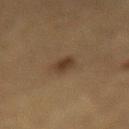Part of a total-body skin-imaging series; this lesion was reviewed on a skin check and was not flagged for biopsy. Imaged with cross-polarized lighting. Located on the mid back. Automated tile analysis of the lesion measured an average lesion color of about L≈31 a*≈13 b*≈25 (CIELAB), about 8 CIELAB-L* units darker than the surrounding skin, and a normalized border contrast of about 8. And it measured an automated nevus-likeness rating near 90 out of 100 and a lesion-detection confidence of about 100/100. About 2.5 mm across. A close-up tile cropped from a whole-body skin photograph, about 15 mm across. A male subject, in their mid- to late 80s or older.